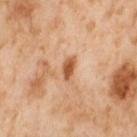Clinical impression:
Part of a total-body skin-imaging series; this lesion was reviewed on a skin check and was not flagged for biopsy.
Background:
The lesion is on the right thigh. A female subject roughly 55 years of age. A lesion tile, about 15 mm wide, cut from a 3D total-body photograph. Captured under cross-polarized illumination. The recorded lesion diameter is about 3 mm.